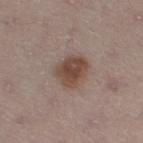Findings:
– notes — no biopsy performed (imaged during a skin exam)
– image-analysis metrics — a shape eccentricity near 0.55 and a shape-asymmetry score of about 0.2 (0 = symmetric); a mean CIELAB color near L≈46 a*≈17 b*≈24, roughly 11 lightness units darker than nearby skin, and a lesion-to-skin contrast of about 9 (normalized; higher = more distinct); internal color variation of about 3.5 on a 0–10 scale and radial color variation of about 1
– lighting — white-light illumination
– subject — male, roughly 65 years of age
– image source — ~15 mm crop, total-body skin-cancer survey
– lesion diameter — about 3.5 mm
– body site — the leg